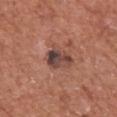The lesion was photographed on a routine skin check and not biopsied; there is no pathology result.
Imaged with white-light lighting.
The patient is a male about 75 years old.
Automated image analysis of the tile measured a border-irregularity rating of about 3/10.
About 3.5 mm across.
On the chest.
A region of skin cropped from a whole-body photographic capture, roughly 15 mm wide.